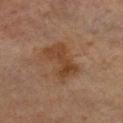biopsy status: total-body-photography surveillance lesion; no biopsy
anatomic site: the left upper arm
image source: ~15 mm crop, total-body skin-cancer survey
automated lesion analysis: a lesion area of about 11 mm², an eccentricity of roughly 0.85, and a shape-asymmetry score of about 0.25 (0 = symmetric); a mean CIELAB color near L≈35 a*≈17 b*≈28, about 7 CIELAB-L* units darker than the surrounding skin, and a normalized lesion–skin contrast near 7.5; border irregularity of about 3.5 on a 0–10 scale, a color-variation rating of about 3/10, and peripheral color asymmetry of about 1
patient: female, approximately 80 years of age